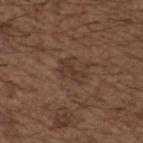Assessment: The lesion was tiled from a total-body skin photograph and was not biopsied. Acquisition and patient details: A male subject, aged around 50. The recorded lesion diameter is about 3.5 mm. The lesion is located on the chest. A lesion tile, about 15 mm wide, cut from a 3D total-body photograph. Captured under white-light illumination.